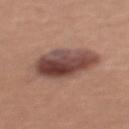- automated lesion analysis — a nevus-likeness score of about 75/100
- image — ~15 mm tile from a whole-body skin photo
- lesion diameter — ≈6 mm
- location — the chest
- illumination — white-light
- patient — female, aged 33 to 37